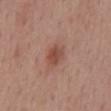Findings:
- workup — imaged on a skin check; not biopsied
- body site — the mid back
- lesion diameter — ~3 mm (longest diameter)
- illumination — white-light
- image — total-body-photography crop, ~15 mm field of view
- automated metrics — a lesion color around L≈48 a*≈23 b*≈27 in CIELAB, roughly 9 lightness units darker than nearby skin, and a normalized lesion–skin contrast near 7
- patient — female, aged approximately 50